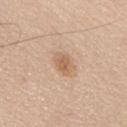follow-up: catalogued during a skin exam; not biopsied
patient: male, aged 63 to 67
lesion size: ~3 mm (longest diameter)
lighting: white-light
anatomic site: the back
image: 15 mm crop, total-body photography
automated lesion analysis: border irregularity of about 2.5 on a 0–10 scale and peripheral color asymmetry of about 1.5; a nevus-likeness score of about 50/100